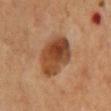<lesion>
<biopsy_status>not biopsied; imaged during a skin examination</biopsy_status>
<patient>
  <sex>male</sex>
  <age_approx>60</age_approx>
</patient>
<image>
  <source>total-body photography crop</source>
  <field_of_view_mm>15</field_of_view_mm>
</image>
<lighting>cross-polarized</lighting>
<site>mid back</site>
</lesion>